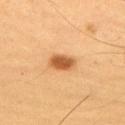This lesion was catalogued during total-body skin photography and was not selected for biopsy. The lesion is on the arm. A male subject, in their mid-50s. Captured under cross-polarized illumination. Cropped from a whole-body photographic skin survey; the tile spans about 15 mm.